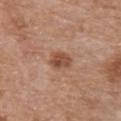This lesion was catalogued during total-body skin photography and was not selected for biopsy.
Automated image analysis of the tile measured a shape eccentricity near 0.75 and a symmetry-axis asymmetry near 0.25. The analysis additionally found an average lesion color of about L≈49 a*≈22 b*≈30 (CIELAB), a lesion–skin lightness drop of about 11, and a normalized lesion–skin contrast near 8.
A male subject, aged approximately 65.
A 15 mm close-up tile from a total-body photography series done for melanoma screening.
The lesion is on the chest.
This is a white-light tile.